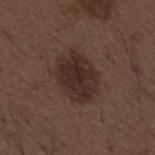This lesion was catalogued during total-body skin photography and was not selected for biopsy.
Approximately 5.5 mm at its widest.
A 15 mm crop from a total-body photograph taken for skin-cancer surveillance.
A male patient, in their 50s.
Captured under white-light illumination.
The lesion is on the mid back.